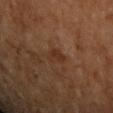Q: Is there a histopathology result?
A: catalogued during a skin exam; not biopsied
Q: What are the patient's age and sex?
A: male, in their mid- to late 50s
Q: What is the anatomic site?
A: the left upper arm
Q: Illumination type?
A: cross-polarized
Q: What is the imaging modality?
A: ~15 mm tile from a whole-body skin photo
Q: Lesion size?
A: about 2.5 mm